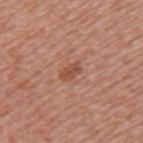{
  "biopsy_status": "not biopsied; imaged during a skin examination",
  "patient": {
    "sex": "female",
    "age_approx": 45
  },
  "lighting": "white-light",
  "image": {
    "source": "total-body photography crop",
    "field_of_view_mm": 15
  },
  "lesion_size": {
    "long_diameter_mm_approx": 2.5
  },
  "site": "arm"
}